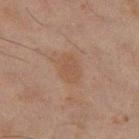  biopsy_status: not biopsied; imaged during a skin examination
  lesion_size:
    long_diameter_mm_approx: 3.5
  automated_metrics:
    border_irregularity_0_10: 2.0
    color_variation_0_10: 2.0
    peripheral_color_asymmetry: 1.0
  site: left thigh
  lighting: cross-polarized
  patient:
    sex: male
    age_approx: 45
  image:
    source: total-body photography crop
    field_of_view_mm: 15